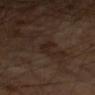This lesion was catalogued during total-body skin photography and was not selected for biopsy. The tile uses cross-polarized illumination. The lesion is on the left forearm. About 3.5 mm across. A male subject about 65 years old. A roughly 15 mm field-of-view crop from a total-body skin photograph.